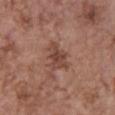Q: Was a biopsy performed?
A: no biopsy performed (imaged during a skin exam)
Q: What are the patient's age and sex?
A: female, aged around 65
Q: Where on the body is the lesion?
A: the chest
Q: What kind of image is this?
A: ~15 mm crop, total-body skin-cancer survey
Q: Automated lesion metrics?
A: a footprint of about 7.5 mm², an outline eccentricity of about 0.8 (0 = round, 1 = elongated), and a symmetry-axis asymmetry near 0.4; a border-irregularity index near 4.5/10; an automated nevus-likeness rating near 0 out of 100 and a lesion-detection confidence of about 100/100
Q: What is the lesion's diameter?
A: ~4 mm (longest diameter)